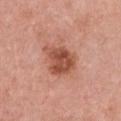biopsy_status: not biopsied; imaged during a skin examination
patient:
  sex: female
  age_approx: 40
image:
  source: total-body photography crop
  field_of_view_mm: 15
automated_metrics:
  cielab_L: 51
  cielab_a: 27
  cielab_b: 31
  vs_skin_darker_L: 13.0
  vs_skin_contrast_norm: 9.0
  nevus_likeness_0_100: 85
  lesion_detection_confidence_0_100: 100
site: front of the torso